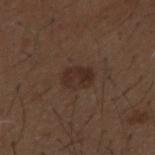<case>
<biopsy_status>not biopsied; imaged during a skin examination</biopsy_status>
<automated_metrics>
  <color_variation_0_10>3.0</color_variation_0_10>
  <peripheral_color_asymmetry>1.0</peripheral_color_asymmetry>
</automated_metrics>
<image>
  <source>total-body photography crop</source>
  <field_of_view_mm>15</field_of_view_mm>
</image>
<patient>
  <sex>male</sex>
  <age_approx>50</age_approx>
</patient>
<lesion_size>
  <long_diameter_mm_approx>3.5</long_diameter_mm_approx>
</lesion_size>
<site>mid back</site>
</case>